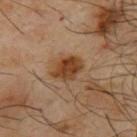Background: A roughly 15 mm field-of-view crop from a total-body skin photograph. A male patient, aged approximately 65. The lesion is located on the back. Approximately 3.5 mm at its widest.